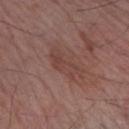Background:
The patient is a male approximately 65 years of age. Located on the right forearm. A region of skin cropped from a whole-body photographic capture, roughly 15 mm wide.